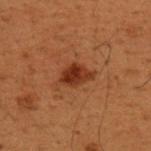| feature | finding |
|---|---|
| workup | catalogued during a skin exam; not biopsied |
| lighting | cross-polarized illumination |
| site | the upper back |
| image | ~15 mm tile from a whole-body skin photo |
| patient | male, aged around 50 |
| size | ≈3.5 mm |